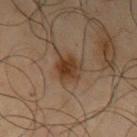  biopsy_status: not biopsied; imaged during a skin examination
  automated_metrics:
    area_mm2_approx: 8.0
    eccentricity: 0.5
    shape_asymmetry: 0.2
    cielab_L: 36
    cielab_a: 17
    cielab_b: 28
    vs_skin_darker_L: 9.0
    vs_skin_contrast_norm: 9.0
    border_irregularity_0_10: 2.0
    color_variation_0_10: 4.5
    peripheral_color_asymmetry: 1.5
    lesion_detection_confidence_0_100: 100
  patient:
    sex: male
    age_approx: 65
  lighting: cross-polarized
  image:
    source: total-body photography crop
    field_of_view_mm: 15
  site: left upper arm
  lesion_size:
    long_diameter_mm_approx: 3.5